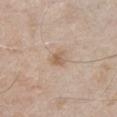Q: Is there a histopathology result?
A: total-body-photography surveillance lesion; no biopsy
Q: What did automated image analysis measure?
A: an outline eccentricity of about 0.6 (0 = round, 1 = elongated) and two-axis asymmetry of about 0.25; a border-irregularity rating of about 2/10, a within-lesion color-variation index near 3/10, and peripheral color asymmetry of about 1
Q: What is the imaging modality?
A: ~15 mm crop, total-body skin-cancer survey
Q: Illumination type?
A: white-light
Q: Who is the patient?
A: male, aged 53 to 57
Q: Lesion size?
A: ~2.5 mm (longest diameter)
Q: What is the anatomic site?
A: the right upper arm